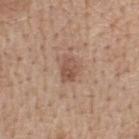  biopsy_status: not biopsied; imaged during a skin examination
  image:
    source: total-body photography crop
    field_of_view_mm: 15
  lighting: white-light
  site: back
  automated_metrics:
    border_irregularity_0_10: 2.5
    color_variation_0_10: 4.0
    lesion_detection_confidence_0_100: 100
  lesion_size:
    long_diameter_mm_approx: 3.0
  patient:
    sex: female
    age_approx: 55